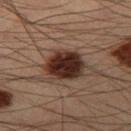Clinical summary: A 15 mm close-up tile from a total-body photography series done for melanoma screening. From the leg. The recorded lesion diameter is about 4 mm. The patient is a male aged around 55.